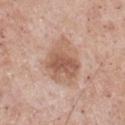Q: What kind of image is this?
A: ~15 mm tile from a whole-body skin photo
Q: How was the tile lit?
A: white-light illumination
Q: Automated lesion metrics?
A: an area of roughly 19 mm², an outline eccentricity of about 0.7 (0 = round, 1 = elongated), and two-axis asymmetry of about 0.25; border irregularity of about 2.5 on a 0–10 scale, a color-variation rating of about 5/10, and a peripheral color-asymmetry measure near 1; a classifier nevus-likeness of about 40/100 and a lesion-detection confidence of about 100/100
Q: Where on the body is the lesion?
A: the chest
Q: Patient demographics?
A: male, aged approximately 55
Q: What is the lesion's diameter?
A: ≈6.5 mm